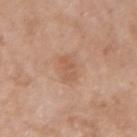Findings:
- biopsy status: catalogued during a skin exam; not biopsied
- lesion diameter: about 3 mm
- automated lesion analysis: a border-irregularity rating of about 3/10, a within-lesion color-variation index near 2/10, and radial color variation of about 0.5; a classifier nevus-likeness of about 15/100 and a lesion-detection confidence of about 100/100
- patient: female, aged approximately 60
- acquisition: 15 mm crop, total-body photography
- location: the left forearm